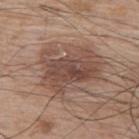Impression: The lesion was photographed on a routine skin check and not biopsied; there is no pathology result. Clinical summary: A male patient, aged around 70. Captured under white-light illumination. Approximately 6.5 mm at its widest. A roughly 15 mm field-of-view crop from a total-body skin photograph. Located on the upper back. The lesion-visualizer software estimated a symmetry-axis asymmetry near 0.35. And it measured a lesion color around L≈47 a*≈18 b*≈25 in CIELAB, roughly 10 lightness units darker than nearby skin, and a normalized border contrast of about 7.5. The analysis additionally found a border-irregularity index near 6/10, a color-variation rating of about 5.5/10, and peripheral color asymmetry of about 2.